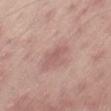Clinical impression: Recorded during total-body skin imaging; not selected for excision or biopsy. Clinical summary: Imaged with white-light lighting. About 3.5 mm across. The lesion is located on the right thigh. A 15 mm close-up extracted from a 3D total-body photography capture. A male patient in their mid- to late 60s.